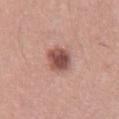notes = no biopsy performed (imaged during a skin exam)
site = the right thigh
subject = female, roughly 40 years of age
diameter = ≈4 mm
image = ~15 mm tile from a whole-body skin photo